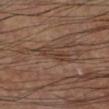Q: Automated lesion metrics?
A: an area of roughly 6 mm², an outline eccentricity of about 0.9 (0 = round, 1 = elongated), and two-axis asymmetry of about 0.3; a lesion color around L≈37 a*≈16 b*≈25 in CIELAB, a lesion–skin lightness drop of about 7, and a normalized border contrast of about 6.5; border irregularity of about 3.5 on a 0–10 scale, a color-variation rating of about 3/10, and a peripheral color-asymmetry measure near 1.5
Q: Patient demographics?
A: male, aged approximately 60
Q: Lesion location?
A: the left lower leg
Q: Lesion size?
A: ~4 mm (longest diameter)
Q: How was the tile lit?
A: cross-polarized illumination
Q: What is the imaging modality?
A: 15 mm crop, total-body photography